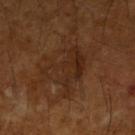follow-up: no biopsy performed (imaged during a skin exam)
body site: the right upper arm
lesion diameter: ~6 mm (longest diameter)
acquisition: 15 mm crop, total-body photography
lighting: cross-polarized illumination
subject: male, in their mid- to late 60s